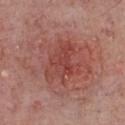Case summary:
- workup — catalogued during a skin exam; not biopsied
- image — 15 mm crop, total-body photography
- patient — male, in their mid-70s
- anatomic site — the chest